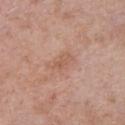Background:
A 15 mm close-up extracted from a 3D total-body photography capture. The recorded lesion diameter is about 3 mm. Captured under white-light illumination. The patient is a female in their mid- to late 50s. On the right lower leg.